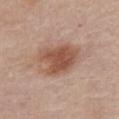Clinical impression:
Imaged during a routine full-body skin examination; the lesion was not biopsied and no histopathology is available.
Acquisition and patient details:
A region of skin cropped from a whole-body photographic capture, roughly 15 mm wide. From the abdomen. Captured under white-light illumination. The lesion's longest dimension is about 5.5 mm. A female patient, aged 63 to 67.